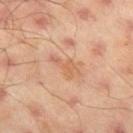This lesion was catalogued during total-body skin photography and was not selected for biopsy. The lesion's longest dimension is about 3.5 mm. This image is a 15 mm lesion crop taken from a total-body photograph. Located on the left thigh. The lesion-visualizer software estimated a footprint of about 4 mm² and a shape-asymmetry score of about 0.55 (0 = symmetric). The analysis additionally found an average lesion color of about L≈61 a*≈23 b*≈35 (CIELAB) and a lesion–skin lightness drop of about 7. The analysis additionally found border irregularity of about 6.5 on a 0–10 scale, a within-lesion color-variation index near 1/10, and a peripheral color-asymmetry measure near 0.5. And it measured a detector confidence of about 100 out of 100 that the crop contains a lesion. Imaged with cross-polarized lighting. A male subject aged 43–47.